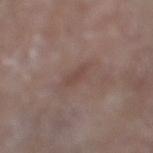The lesion was photographed on a routine skin check and not biopsied; there is no pathology result.
On the right lower leg.
Imaged with white-light lighting.
A roughly 15 mm field-of-view crop from a total-body skin photograph.
The patient is a male in their 80s.
Approximately 3 mm at its widest.